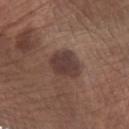{"biopsy_status": "not biopsied; imaged during a skin examination", "site": "left forearm", "automated_metrics": {"cielab_L": 37, "cielab_a": 17, "cielab_b": 20, "vs_skin_darker_L": 10.0, "vs_skin_contrast_norm": 9.5, "border_irregularity_0_10": 2.0, "color_variation_0_10": 2.5, "peripheral_color_asymmetry": 0.5, "nevus_likeness_0_100": 55, "lesion_detection_confidence_0_100": 100}, "image": {"source": "total-body photography crop", "field_of_view_mm": 15}, "patient": {"sex": "male", "age_approx": 65}}